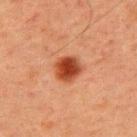Q: Is there a histopathology result?
A: catalogued during a skin exam; not biopsied
Q: What are the patient's age and sex?
A: male, aged 58–62
Q: Where on the body is the lesion?
A: the upper back
Q: What kind of image is this?
A: ~15 mm crop, total-body skin-cancer survey